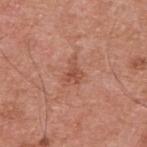Q: Was a biopsy performed?
A: total-body-photography surveillance lesion; no biopsy
Q: What is the lesion's diameter?
A: about 3.5 mm
Q: Who is the patient?
A: male, aged 53 to 57
Q: How was the tile lit?
A: white-light
Q: What is the imaging modality?
A: 15 mm crop, total-body photography
Q: What is the anatomic site?
A: the upper back